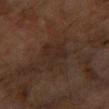Q: Was this lesion biopsied?
A: imaged on a skin check; not biopsied
Q: What is the imaging modality?
A: ~15 mm tile from a whole-body skin photo
Q: Lesion location?
A: the right forearm
Q: What lighting was used for the tile?
A: cross-polarized illumination
Q: How large is the lesion?
A: ~4.5 mm (longest diameter)
Q: Patient demographics?
A: female, roughly 60 years of age